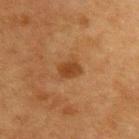Assessment:
This lesion was catalogued during total-body skin photography and was not selected for biopsy.
Clinical summary:
Located on the upper back. An algorithmic analysis of the crop reported an automated nevus-likeness rating near 90 out of 100 and a detector confidence of about 100 out of 100 that the crop contains a lesion. A male patient, roughly 75 years of age. This is a cross-polarized tile. Approximately 2.5 mm at its widest. Cropped from a total-body skin-imaging series; the visible field is about 15 mm.